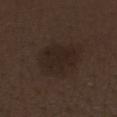Impression: Captured during whole-body skin photography for melanoma surveillance; the lesion was not biopsied. Acquisition and patient details: A 15 mm close-up tile from a total-body photography series done for melanoma screening. Approximately 4.5 mm at its widest. The subject is a male about 70 years old. From the left forearm. Imaged with white-light lighting.